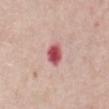Imaged during a routine full-body skin examination; the lesion was not biopsied and no histopathology is available. An algorithmic analysis of the crop reported border irregularity of about 2 on a 0–10 scale, a within-lesion color-variation index near 3.5/10, and peripheral color asymmetry of about 1. A female subject aged approximately 65. A 15 mm close-up extracted from a 3D total-body photography capture. Captured under white-light illumination. The lesion's longest dimension is about 3 mm. Located on the chest.